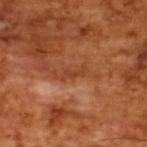A male patient about 65 years old. Cropped from a total-body skin-imaging series; the visible field is about 15 mm.